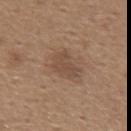The lesion was tiled from a total-body skin photograph and was not biopsied. The lesion is located on the back. Automated tile analysis of the lesion measured a mean CIELAB color near L≈47 a*≈17 b*≈27 and a normalized border contrast of about 6. It also reported a nevus-likeness score of about 5/100 and a detector confidence of about 100 out of 100 that the crop contains a lesion. A male subject aged 63 to 67. Measured at roughly 3.5 mm in maximum diameter. A close-up tile cropped from a whole-body skin photograph, about 15 mm across. This is a white-light tile.